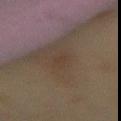follow-up = catalogued during a skin exam; not biopsied | site = the lower back | imaging modality = 15 mm crop, total-body photography | diameter = ≈2.5 mm | tile lighting = cross-polarized illumination | patient = female, roughly 60 years of age.